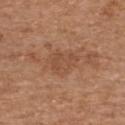Findings:
• follow-up: total-body-photography surveillance lesion; no biopsy
• lesion size: ≈3.5 mm
• acquisition: ~15 mm crop, total-body skin-cancer survey
• subject: male, in their 70s
• automated lesion analysis: a border-irregularity index near 4/10; an automated nevus-likeness rating near 0 out of 100 and lesion-presence confidence of about 100/100
• illumination: white-light illumination
• location: the upper back